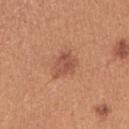Q: Is there a histopathology result?
A: catalogued during a skin exam; not biopsied
Q: How large is the lesion?
A: ≈3 mm
Q: How was this image acquired?
A: ~15 mm crop, total-body skin-cancer survey
Q: Who is the patient?
A: female, aged approximately 40
Q: How was the tile lit?
A: white-light illumination
Q: Lesion location?
A: the right upper arm